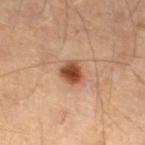Assessment:
Imaged during a routine full-body skin examination; the lesion was not biopsied and no histopathology is available.
Context:
The patient is a male approximately 45 years of age. From the left lower leg. A 15 mm crop from a total-body photograph taken for skin-cancer surveillance.